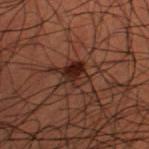Impression:
This lesion was catalogued during total-body skin photography and was not selected for biopsy.
Context:
Automated tile analysis of the lesion measured a footprint of about 6.5 mm² and a shape eccentricity near 0.45. The software also gave a border-irregularity rating of about 2.5/10, internal color variation of about 6.5 on a 0–10 scale, and a peripheral color-asymmetry measure near 2.5. A region of skin cropped from a whole-body photographic capture, roughly 15 mm wide. The patient is a male aged approximately 50. Captured under cross-polarized illumination. Longest diameter approximately 3 mm. From the leg.